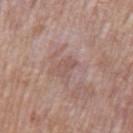Findings:
* follow-up: total-body-photography surveillance lesion; no biopsy
* lesion size: about 2.5 mm
* illumination: white-light illumination
* anatomic site: the left upper arm
* acquisition: 15 mm crop, total-body photography
* automated metrics: a footprint of about 3.5 mm² and an eccentricity of roughly 0.85; a border-irregularity rating of about 3.5/10, a color-variation rating of about 1.5/10, and radial color variation of about 0.5; lesion-presence confidence of about 95/100
* patient: male, aged 68 to 72